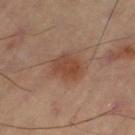Captured during whole-body skin photography for melanoma surveillance; the lesion was not biopsied. Located on the left thigh. A 15 mm close-up tile from a total-body photography series done for melanoma screening.